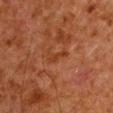Q: Was a biopsy performed?
A: no biopsy performed (imaged during a skin exam)
Q: Who is the patient?
A: male, aged around 60
Q: What is the anatomic site?
A: the front of the torso
Q: What is the imaging modality?
A: ~15 mm crop, total-body skin-cancer survey
Q: What is the lesion's diameter?
A: about 3 mm
Q: Automated lesion metrics?
A: a footprint of about 2.5 mm², an eccentricity of roughly 0.95, and a shape-asymmetry score of about 0.45 (0 = symmetric); a nevus-likeness score of about 0/100
Q: Illumination type?
A: cross-polarized illumination